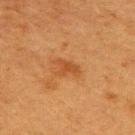Impression: The lesion was photographed on a routine skin check and not biopsied; there is no pathology result. Context: A 15 mm crop from a total-body photograph taken for skin-cancer surveillance. The recorded lesion diameter is about 3 mm. On the upper back. A female patient, about 40 years old.